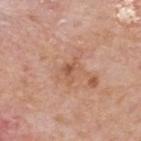follow-up = catalogued during a skin exam; not biopsied
imaging modality = 15 mm crop, total-body photography
subject = male, roughly 75 years of age
lesion diameter = about 3 mm
anatomic site = the upper back